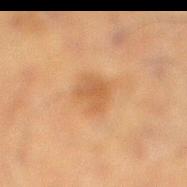{
  "biopsy_status": "not biopsied; imaged during a skin examination",
  "lesion_size": {
    "long_diameter_mm_approx": 3.5
  },
  "patient": {
    "sex": "male",
    "age_approx": 60
  },
  "lighting": "cross-polarized",
  "site": "leg",
  "automated_metrics": {
    "area_mm2_approx": 7.5,
    "eccentricity": 0.6,
    "shape_asymmetry": 0.25,
    "nevus_likeness_0_100": 15,
    "lesion_detection_confidence_0_100": 100
  },
  "image": {
    "source": "total-body photography crop",
    "field_of_view_mm": 15
  }
}